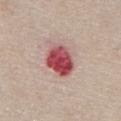| key | value |
|---|---|
| notes | no biopsy performed (imaged during a skin exam) |
| lesion size | ≈4.5 mm |
| illumination | white-light |
| image-analysis metrics | an outline eccentricity of about 0.6 (0 = round, 1 = elongated) and a symmetry-axis asymmetry near 0.15; a border-irregularity rating of about 1.5/10 and peripheral color asymmetry of about 2 |
| patient | male, approximately 80 years of age |
| anatomic site | the chest |
| imaging modality | ~15 mm crop, total-body skin-cancer survey |